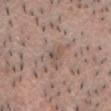Assessment:
Captured during whole-body skin photography for melanoma surveillance; the lesion was not biopsied.
Context:
Automated image analysis of the tile measured an outline eccentricity of about 0.8 (0 = round, 1 = elongated). The analysis additionally found a classifier nevus-likeness of about 0/100 and a lesion-detection confidence of about 55/100. This is a white-light tile. Cropped from a whole-body photographic skin survey; the tile spans about 15 mm. On the chest. A male subject about 50 years old. Measured at roughly 3 mm in maximum diameter.